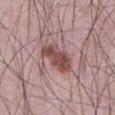Clinical impression:
Imaged during a routine full-body skin examination; the lesion was not biopsied and no histopathology is available.
Image and clinical context:
Imaged with white-light lighting. The patient is a male in their mid- to late 60s. A 15 mm close-up extracted from a 3D total-body photography capture. From the abdomen.